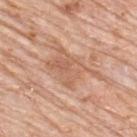biopsy status: no biopsy performed (imaged during a skin exam) | automated metrics: a shape-asymmetry score of about 0.4 (0 = symmetric); a lesion color around L≈60 a*≈22 b*≈32 in CIELAB, a lesion–skin lightness drop of about 8, and a normalized border contrast of about 5.5; a lesion-detection confidence of about 80/100 | acquisition: ~15 mm crop, total-body skin-cancer survey | location: the upper back | subject: male, roughly 80 years of age | lesion diameter: ~6 mm (longest diameter) | illumination: white-light.